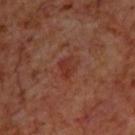workup: catalogued during a skin exam; not biopsied | imaging modality: total-body-photography crop, ~15 mm field of view | location: the back | subject: male, aged approximately 70 | lighting: cross-polarized | image-analysis metrics: a lesion area of about 4.5 mm², an outline eccentricity of about 0.65 (0 = round, 1 = elongated), and a shape-asymmetry score of about 0.3 (0 = symmetric); a border-irregularity rating of about 3/10, a color-variation rating of about 3/10, and radial color variation of about 1; a classifier nevus-likeness of about 0/100 and a detector confidence of about 100 out of 100 that the crop contains a lesion.